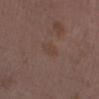<lesion>
<biopsy_status>not biopsied; imaged during a skin examination</biopsy_status>
<lighting>white-light</lighting>
<image>
  <source>total-body photography crop</source>
  <field_of_view_mm>15</field_of_view_mm>
</image>
<site>right lower leg</site>
<patient>
  <sex>female</sex>
  <age_approx>35</age_approx>
</patient>
</lesion>